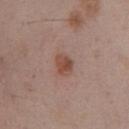Clinical impression:
Imaged during a routine full-body skin examination; the lesion was not biopsied and no histopathology is available.
Acquisition and patient details:
A close-up tile cropped from a whole-body skin photograph, about 15 mm across. Captured under white-light illumination. The subject is a male roughly 65 years of age. Measured at roughly 3 mm in maximum diameter. Automated tile analysis of the lesion measured an area of roughly 5 mm², a shape eccentricity near 0.7, and two-axis asymmetry of about 0.2. The software also gave an average lesion color of about L≈48 a*≈21 b*≈27 (CIELAB), roughly 9 lightness units darker than nearby skin, and a normalized border contrast of about 8. It also reported a nevus-likeness score of about 90/100 and a lesion-detection confidence of about 100/100. The lesion is on the chest.